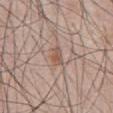A region of skin cropped from a whole-body photographic capture, roughly 15 mm wide.
Automated tile analysis of the lesion measured an average lesion color of about L≈55 a*≈19 b*≈27 (CIELAB), roughly 8 lightness units darker than nearby skin, and a lesion-to-skin contrast of about 6.5 (normalized; higher = more distinct).
A male patient, aged around 55.
Imaged with white-light lighting.
The lesion's longest dimension is about 2.5 mm.
On the front of the torso.